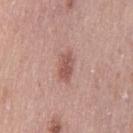Context:
A roughly 15 mm field-of-view crop from a total-body skin photograph. Approximately 3.5 mm at its widest. A female patient, roughly 40 years of age. From the left thigh.